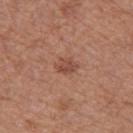Part of a total-body skin-imaging series; this lesion was reviewed on a skin check and was not flagged for biopsy.
Cropped from a whole-body photographic skin survey; the tile spans about 15 mm.
The lesion is on the left thigh.
This is a white-light tile.
A female patient in their 40s.
The lesion-visualizer software estimated a mean CIELAB color near L≈48 a*≈23 b*≈28 and a normalized lesion–skin contrast near 6.5. The software also gave border irregularity of about 1.5 on a 0–10 scale and radial color variation of about 1.